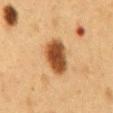<lesion>
<biopsy_status>not biopsied; imaged during a skin examination</biopsy_status>
<site>front of the torso</site>
<lighting>cross-polarized</lighting>
<patient>
  <sex>male</sex>
  <age_approx>55</age_approx>
</patient>
<lesion_size>
  <long_diameter_mm_approx>4.5</long_diameter_mm_approx>
</lesion_size>
<image>
  <source>total-body photography crop</source>
  <field_of_view_mm>15</field_of_view_mm>
</image>
</lesion>